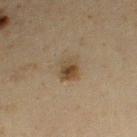Image and clinical context:
A male subject about 60 years old. Approximately 3 mm at its widest. Imaged with cross-polarized lighting. This image is a 15 mm lesion crop taken from a total-body photograph. On the right upper arm. The lesion-visualizer software estimated an area of roughly 6 mm², a shape eccentricity near 0.6, and a symmetry-axis asymmetry near 0.2. And it measured a border-irregularity rating of about 2/10, a color-variation rating of about 5.5/10, and a peripheral color-asymmetry measure near 2. And it measured an automated nevus-likeness rating near 85 out of 100 and lesion-presence confidence of about 100/100.